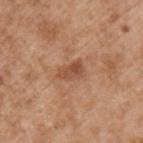follow-up: imaged on a skin check; not biopsied
diameter: ≈3 mm
site: the left upper arm
TBP lesion metrics: an area of roughly 5 mm², a shape eccentricity near 0.85, and a shape-asymmetry score of about 0.25 (0 = symmetric); a lesion color around L≈50 a*≈23 b*≈33 in CIELAB, roughly 9 lightness units darker than nearby skin, and a normalized border contrast of about 7; lesion-presence confidence of about 100/100
patient: male, aged 53–57
lighting: white-light
image: ~15 mm crop, total-body skin-cancer survey